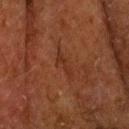Captured during whole-body skin photography for melanoma surveillance; the lesion was not biopsied. Longest diameter approximately 3.5 mm. The lesion is on the head or neck. A male patient, approximately 70 years of age. An algorithmic analysis of the crop reported a lesion color around L≈26 a*≈21 b*≈27 in CIELAB and a lesion–skin lightness drop of about 5. The analysis additionally found border irregularity of about 6.5 on a 0–10 scale, a color-variation rating of about 0/10, and peripheral color asymmetry of about 0. The tile uses cross-polarized illumination. Cropped from a total-body skin-imaging series; the visible field is about 15 mm.